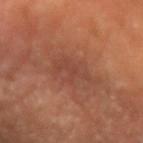Captured during whole-body skin photography for melanoma surveillance; the lesion was not biopsied. The tile uses cross-polarized illumination. The recorded lesion diameter is about 5.5 mm. A 15 mm crop from a total-body photograph taken for skin-cancer surveillance. A female patient, aged 63–67. Located on the left forearm.